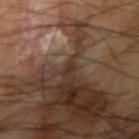This lesion was catalogued during total-body skin photography and was not selected for biopsy. A male patient aged approximately 65. This image is a 15 mm lesion crop taken from a total-body photograph. Imaged with cross-polarized lighting. An algorithmic analysis of the crop reported a lesion color around L≈31 a*≈16 b*≈24 in CIELAB, about 7 CIELAB-L* units darker than the surrounding skin, and a normalized border contrast of about 7. And it measured an automated nevus-likeness rating near 0 out of 100 and a detector confidence of about 50 out of 100 that the crop contains a lesion. The lesion is on the right upper arm.